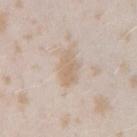Imaged during a routine full-body skin examination; the lesion was not biopsied and no histopathology is available.
A lesion tile, about 15 mm wide, cut from a 3D total-body photograph.
Automated tile analysis of the lesion measured a lesion area of about 7 mm² and a shape eccentricity near 0.85. The software also gave a border-irregularity index near 2.5/10, internal color variation of about 2 on a 0–10 scale, and radial color variation of about 0.5. And it measured a classifier nevus-likeness of about 0/100 and a lesion-detection confidence of about 85/100.
A female subject, in their mid- to late 20s.
Imaged with white-light lighting.
The lesion is on the right upper arm.